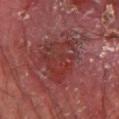Imaged during a routine full-body skin examination; the lesion was not biopsied and no histopathology is available.
The lesion is on the left thigh.
A roughly 15 mm field-of-view crop from a total-body skin photograph.
A male subject, in their mid- to late 50s.